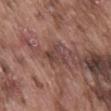{"biopsy_status": "not biopsied; imaged during a skin examination", "lesion_size": {"long_diameter_mm_approx": 3.0}, "site": "mid back", "automated_metrics": {"area_mm2_approx": 5.5, "eccentricity": 0.65, "shape_asymmetry": 0.4, "border_irregularity_0_10": 4.0, "color_variation_0_10": 3.5, "peripheral_color_asymmetry": 1.0, "nevus_likeness_0_100": 0, "lesion_detection_confidence_0_100": 90}, "image": {"source": "total-body photography crop", "field_of_view_mm": 15}, "lighting": "white-light", "patient": {"sex": "male", "age_approx": 75}}